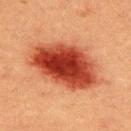lighting: cross-polarized
site: upper back
patient:
  sex: male
  age_approx: 40
lesion_size:
  long_diameter_mm_approx: 9.0
automated_metrics:
  border_irregularity_0_10: 2.0
  color_variation_0_10: 7.0
  peripheral_color_asymmetry: 2.0
  nevus_likeness_0_100: 100
  lesion_detection_confidence_0_100: 100
image:
  source: total-body photography crop
  field_of_view_mm: 15